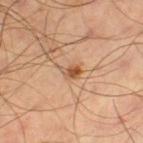<lesion>
<biopsy_status>not biopsied; imaged during a skin examination</biopsy_status>
<site>left thigh</site>
<automated_metrics>
  <area_mm2_approx>3.0</area_mm2_approx>
  <eccentricity>0.7</eccentricity>
  <shape_asymmetry>0.3</shape_asymmetry>
  <border_irregularity_0_10>2.5</border_irregularity_0_10>
  <color_variation_0_10>3.5</color_variation_0_10>
  <peripheral_color_asymmetry>1.0</peripheral_color_asymmetry>
  <nevus_likeness_0_100>95</nevus_likeness_0_100>
  <lesion_detection_confidence_0_100>100</lesion_detection_confidence_0_100>
</automated_metrics>
<lesion_size>
  <long_diameter_mm_approx>2.5</long_diameter_mm_approx>
</lesion_size>
<lighting>cross-polarized</lighting>
<patient>
  <sex>male</sex>
  <age_approx>55</age_approx>
</patient>
<image>
  <source>total-body photography crop</source>
  <field_of_view_mm>15</field_of_view_mm>
</image>
</lesion>